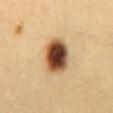biopsy status: catalogued during a skin exam; not biopsied | diameter: ~4.5 mm (longest diameter) | lighting: cross-polarized | patient: female, aged 28–32 | image: ~15 mm crop, total-body skin-cancer survey | TBP lesion metrics: a mean CIELAB color near L≈42 a*≈19 b*≈31 and a lesion–skin lightness drop of about 24; a border-irregularity rating of about 1/10, a within-lesion color-variation index near 8/10, and a peripheral color-asymmetry measure near 2; a classifier nevus-likeness of about 100/100 and a detector confidence of about 100 out of 100 that the crop contains a lesion | body site: the mid back.